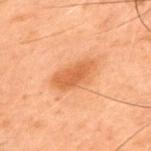follow-up = no biopsy performed (imaged during a skin exam) | image source = total-body-photography crop, ~15 mm field of view | tile lighting = cross-polarized | patient = male, about 50 years old | diameter = ~5 mm (longest diameter) | site = the upper back.